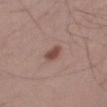workup=catalogued during a skin exam; not biopsied
body site=the right thigh
patient=male, aged around 40
imaging modality=~15 mm tile from a whole-body skin photo
lighting=white-light
image-analysis metrics=an automated nevus-likeness rating near 95 out of 100 and a lesion-detection confidence of about 100/100
diameter=≈2.5 mm